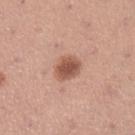Assessment: Part of a total-body skin-imaging series; this lesion was reviewed on a skin check and was not flagged for biopsy. Context: Captured under white-light illumination. On the left lower leg. A female patient, aged 28 to 32. An algorithmic analysis of the crop reported a lesion area of about 7 mm². Cropped from a total-body skin-imaging series; the visible field is about 15 mm.